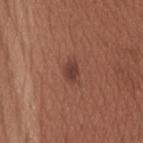Q: What are the patient's age and sex?
A: male, aged 33–37
Q: Automated lesion metrics?
A: border irregularity of about 2 on a 0–10 scale and radial color variation of about 0.5
Q: Lesion location?
A: the head or neck
Q: What is the imaging modality?
A: total-body-photography crop, ~15 mm field of view
Q: Lesion size?
A: ~2.5 mm (longest diameter)
Q: How was the tile lit?
A: white-light